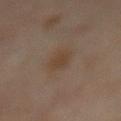* follow-up — no biopsy performed (imaged during a skin exam)
* tile lighting — cross-polarized
* size — about 2.5 mm
* site — the mid back
* image source — total-body-photography crop, ~15 mm field of view
* automated lesion analysis — a lesion–skin lightness drop of about 6 and a lesion-to-skin contrast of about 6.5 (normalized; higher = more distinct); a border-irregularity index near 2/10 and radial color variation of about 0.5
* subject — male, about 65 years old